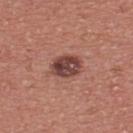  biopsy_status: not biopsied; imaged during a skin examination
  image:
    source: total-body photography crop
    field_of_view_mm: 15
  site: upper back
  lighting: white-light
  automated_metrics:
    area_mm2_approx: 8.0
    eccentricity: 0.65
    shape_asymmetry: 0.15
    border_irregularity_0_10: 1.5
    color_variation_0_10: 5.5
    peripheral_color_asymmetry: 2.0
  patient:
    sex: male
    age_approx: 40
  lesion_size:
    long_diameter_mm_approx: 3.5